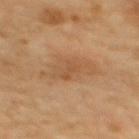notes = no biopsy performed (imaged during a skin exam) | automated metrics = a footprint of about 6.5 mm², a shape eccentricity near 0.9, and a symmetry-axis asymmetry near 0.3; a border-irregularity index near 4/10, internal color variation of about 2.5 on a 0–10 scale, and a peripheral color-asymmetry measure near 1; an automated nevus-likeness rating near 5 out of 100 and a lesion-detection confidence of about 100/100 | anatomic site = the back | acquisition = ~15 mm crop, total-body skin-cancer survey | tile lighting = cross-polarized | patient = female, in their mid- to late 60s | size = ≈4.5 mm.